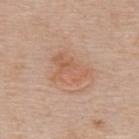Notes:
- workup — catalogued during a skin exam; not biopsied
- lighting — white-light
- imaging modality — ~15 mm tile from a whole-body skin photo
- patient — female, approximately 50 years of age
- location — the upper back
- lesion diameter — ~4.5 mm (longest diameter)